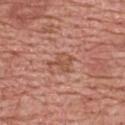{"biopsy_status": "not biopsied; imaged during a skin examination", "lesion_size": {"long_diameter_mm_approx": 3.0}, "automated_metrics": {"color_variation_0_10": 2.5, "peripheral_color_asymmetry": 1.0}, "patient": {"sex": "male", "age_approx": 60}, "image": {"source": "total-body photography crop", "field_of_view_mm": 15}, "lighting": "white-light", "site": "upper back"}